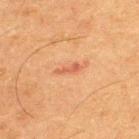Recorded during total-body skin imaging; not selected for excision or biopsy. Imaged with cross-polarized lighting. Automated tile analysis of the lesion measured a mean CIELAB color near L≈46 a*≈25 b*≈30, about 8 CIELAB-L* units darker than the surrounding skin, and a normalized border contrast of about 6.5. A close-up tile cropped from a whole-body skin photograph, about 15 mm across. Measured at roughly 2.5 mm in maximum diameter. The subject is a male in their mid-30s. The lesion is on the upper back.